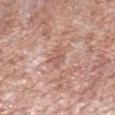Findings:
* biopsy status — catalogued during a skin exam; not biopsied
* site — the right lower leg
* image — ~15 mm tile from a whole-body skin photo
* lighting — white-light
* patient — male, aged 53 to 57